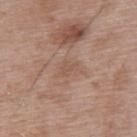Recorded during total-body skin imaging; not selected for excision or biopsy. A lesion tile, about 15 mm wide, cut from a 3D total-body photograph. The recorded lesion diameter is about 2.5 mm. The lesion-visualizer software estimated a lesion color around L≈54 a*≈18 b*≈28 in CIELAB, a lesion–skin lightness drop of about 6, and a normalized border contrast of about 4.5. The software also gave a within-lesion color-variation index near 1.5/10 and peripheral color asymmetry of about 0.5. A male patient, aged 48 to 52. Located on the upper back. The tile uses white-light illumination.